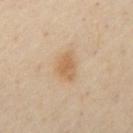Recorded during total-body skin imaging; not selected for excision or biopsy. Located on the chest. A male patient, in their mid-50s. A 15 mm close-up extracted from a 3D total-body photography capture.